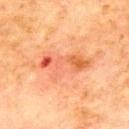Recorded during total-body skin imaging; not selected for excision or biopsy. A male patient aged approximately 70. On the chest. This is a cross-polarized tile. About 6.5 mm across. Automated tile analysis of the lesion measured a shape eccentricity near 0.9 and a shape-asymmetry score of about 0.35 (0 = symmetric). The software also gave a lesion color around L≈54 a*≈28 b*≈35 in CIELAB, about 8 CIELAB-L* units darker than the surrounding skin, and a normalized border contrast of about 6. The software also gave border irregularity of about 4.5 on a 0–10 scale, internal color variation of about 8.5 on a 0–10 scale, and a peripheral color-asymmetry measure near 2.5. Cropped from a total-body skin-imaging series; the visible field is about 15 mm.